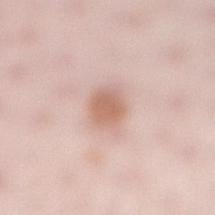notes — catalogued during a skin exam; not biopsied | illumination — white-light | lesion size — about 3 mm | image — ~15 mm crop, total-body skin-cancer survey | patient — female, in their 30s | site — the right lower leg.